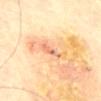Q: Is there a histopathology result?
A: catalogued during a skin exam; not biopsied
Q: What is the anatomic site?
A: the mid back
Q: How large is the lesion?
A: ~3 mm (longest diameter)
Q: What did automated image analysis measure?
A: a border-irregularity index near 4/10, a within-lesion color-variation index near 1/10, and radial color variation of about 0
Q: What is the imaging modality?
A: ~15 mm crop, total-body skin-cancer survey
Q: How was the tile lit?
A: cross-polarized illumination
Q: Who is the patient?
A: male, about 75 years old